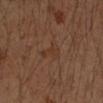<lesion>
  <biopsy_status>not biopsied; imaged during a skin examination</biopsy_status>
  <image>
    <source>total-body photography crop</source>
    <field_of_view_mm>15</field_of_view_mm>
  </image>
  <lighting>cross-polarized</lighting>
  <patient>
    <sex>male</sex>
    <age_approx>50</age_approx>
  </patient>
  <site>left forearm</site>
  <lesion_size>
    <long_diameter_mm_approx>3.0</long_diameter_mm_approx>
  </lesion_size>
</lesion>